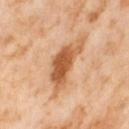biopsy_status: not biopsied; imaged during a skin examination
image:
  source: total-body photography crop
  field_of_view_mm: 15
site: right thigh
automated_metrics:
  area_mm2_approx: 16.0
  eccentricity: 0.9
  shape_asymmetry: 0.5
  cielab_L: 60
  cielab_a: 24
  cielab_b: 39
  vs_skin_darker_L: 14.0
  vs_skin_contrast_norm: 9.0
  color_variation_0_10: 5.5
  peripheral_color_asymmetry: 1.5
  nevus_likeness_0_100: 75
  lesion_detection_confidence_0_100: 100
lighting: cross-polarized
patient:
  sex: female
  age_approx: 55